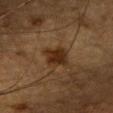The lesion was tiled from a total-body skin photograph and was not biopsied. The lesion-visualizer software estimated a footprint of about 6.5 mm², a shape eccentricity near 0.5, and a symmetry-axis asymmetry near 0.2. And it measured a nevus-likeness score of about 85/100 and a detector confidence of about 100 out of 100 that the crop contains a lesion. This is a cross-polarized tile. The lesion is on the head or neck. Cropped from a total-body skin-imaging series; the visible field is about 15 mm. A female subject about 55 years old.